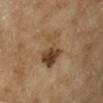biopsy status = catalogued during a skin exam; not biopsied
subject = male, in their mid-60s
site = the leg
lesion diameter = about 4.5 mm
tile lighting = cross-polarized
image = total-body-photography crop, ~15 mm field of view